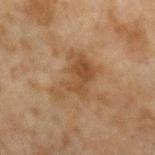No biopsy was performed on this lesion — it was imaged during a full skin examination and was not determined to be concerning. The lesion is on the left forearm. The lesion-visualizer software estimated a footprint of about 14 mm², an eccentricity of roughly 0.75, and a shape-asymmetry score of about 0.4 (0 = symmetric). The analysis additionally found a mean CIELAB color near L≈39 a*≈16 b*≈29, about 7 CIELAB-L* units darker than the surrounding skin, and a lesion-to-skin contrast of about 6.5 (normalized; higher = more distinct). Approximately 5.5 mm at its widest. The patient is a male aged 43 to 47. A 15 mm close-up tile from a total-body photography series done for melanoma screening.